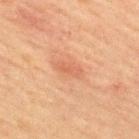Assessment: Recorded during total-body skin imaging; not selected for excision or biopsy. Clinical summary: The lesion is located on the upper back. A male patient, about 60 years old. The total-body-photography lesion software estimated a footprint of about 4.5 mm², an outline eccentricity of about 0.85 (0 = round, 1 = elongated), and two-axis asymmetry of about 0.3. It also reported an average lesion color of about L≈52 a*≈23 b*≈31 (CIELAB), about 6 CIELAB-L* units darker than the surrounding skin, and a normalized border contrast of about 5. A 15 mm close-up extracted from a 3D total-body photography capture. This is a cross-polarized tile. The lesion's longest dimension is about 3.5 mm.